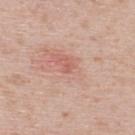| feature | finding |
|---|---|
| image-analysis metrics | an outline eccentricity of about 0.85 (0 = round, 1 = elongated) and a shape-asymmetry score of about 0.3 (0 = symmetric) |
| patient | male, aged approximately 40 |
| anatomic site | the upper back |
| imaging modality | total-body-photography crop, ~15 mm field of view |
| diameter | ~1 mm (longest diameter) |
| tile lighting | white-light illumination |